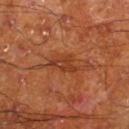Notes:
– notes — total-body-photography surveillance lesion; no biopsy
– acquisition — ~15 mm crop, total-body skin-cancer survey
– site — the right lower leg
– subject — male, approximately 65 years of age